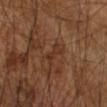Captured during whole-body skin photography for melanoma surveillance; the lesion was not biopsied.
Imaged with cross-polarized lighting.
A lesion tile, about 15 mm wide, cut from a 3D total-body photograph.
The lesion's longest dimension is about 2.5 mm.
On the arm.
A male subject aged approximately 65.